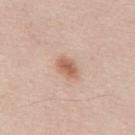workup=total-body-photography surveillance lesion; no biopsy | site=the mid back | subject=male, in their mid-40s | image=~15 mm tile from a whole-body skin photo | illumination=white-light | TBP lesion metrics=a border-irregularity index near 2/10 and radial color variation of about 1.